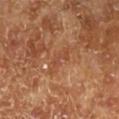workup: no biopsy performed (imaged during a skin exam) | anatomic site: the left lower leg | patient: male, in their 70s | acquisition: total-body-photography crop, ~15 mm field of view | illumination: cross-polarized illumination | automated metrics: a lesion area of about 4.5 mm², an outline eccentricity of about 0.9 (0 = round, 1 = elongated), and a shape-asymmetry score of about 0.5 (0 = symmetric); a lesion color around L≈46 a*≈24 b*≈33 in CIELAB and a normalized border contrast of about 4.5; a classifier nevus-likeness of about 0/100 and a detector confidence of about 75 out of 100 that the crop contains a lesion.